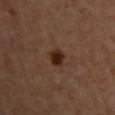<case>
  <biopsy_status>not biopsied; imaged during a skin examination</biopsy_status>
  <site>front of the torso</site>
  <lighting>cross-polarized</lighting>
  <image>
    <source>total-body photography crop</source>
    <field_of_view_mm>15</field_of_view_mm>
  </image>
  <patient>
    <sex>female</sex>
    <age_approx>45</age_approx>
  </patient>
</case>